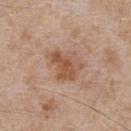Clinical impression:
Captured during whole-body skin photography for melanoma surveillance; the lesion was not biopsied.
Clinical summary:
A male patient in their mid- to late 60s. Cropped from a total-body skin-imaging series; the visible field is about 15 mm. Automated image analysis of the tile measured border irregularity of about 3.5 on a 0–10 scale, internal color variation of about 4 on a 0–10 scale, and radial color variation of about 1. It also reported an automated nevus-likeness rating near 35 out of 100 and lesion-presence confidence of about 100/100. On the chest. About 4.5 mm across. Captured under white-light illumination.